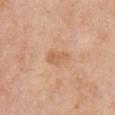{
  "biopsy_status": "not biopsied; imaged during a skin examination",
  "lesion_size": {
    "long_diameter_mm_approx": 3.0
  },
  "automated_metrics": {
    "border_irregularity_0_10": 3.0
  },
  "patient": {
    "sex": "female",
    "age_approx": 55
  },
  "site": "chest",
  "image": {
    "source": "total-body photography crop",
    "field_of_view_mm": 15
  }
}